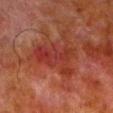biopsy status: no biopsy performed (imaged during a skin exam)
subject: male, aged 78 to 82
anatomic site: the left lower leg
image: ~15 mm tile from a whole-body skin photo
size: ~5.5 mm (longest diameter)